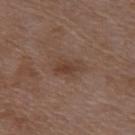| field | value |
|---|---|
| diameter | ~3.5 mm (longest diameter) |
| subject | female, aged 68–72 |
| body site | the upper back |
| automated lesion analysis | a footprint of about 5 mm², an outline eccentricity of about 0.85 (0 = round, 1 = elongated), and a shape-asymmetry score of about 0.3 (0 = symmetric); an automated nevus-likeness rating near 0 out of 100 |
| imaging modality | 15 mm crop, total-body photography |